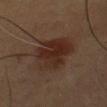The lesion was tiled from a total-body skin photograph and was not biopsied.
Located on the chest.
A region of skin cropped from a whole-body photographic capture, roughly 15 mm wide.
A male subject aged 48–52.